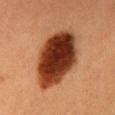The lesion was photographed on a routine skin check and not biopsied; there is no pathology result.
Automated tile analysis of the lesion measured roughly 22 lightness units darker than nearby skin and a lesion-to-skin contrast of about 17 (normalized; higher = more distinct). The analysis additionally found a border-irregularity index near 1.5/10, internal color variation of about 8 on a 0–10 scale, and a peripheral color-asymmetry measure near 2.5. The analysis additionally found a classifier nevus-likeness of about 100/100.
A lesion tile, about 15 mm wide, cut from a 3D total-body photograph.
The recorded lesion diameter is about 9 mm.
Captured under cross-polarized illumination.
The patient is a female roughly 40 years of age.
The lesion is located on the chest.